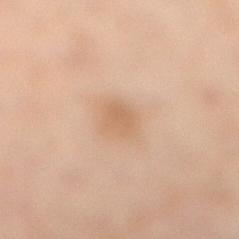follow-up = total-body-photography surveillance lesion; no biopsy
image source = total-body-photography crop, ~15 mm field of view
illumination = cross-polarized illumination
lesion size = ~2.5 mm (longest diameter)
anatomic site = the left leg
patient = female, aged around 55